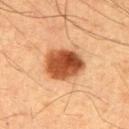The lesion was tiled from a total-body skin photograph and was not biopsied. A roughly 15 mm field-of-view crop from a total-body skin photograph. The lesion's longest dimension is about 5 mm. The lesion is on the arm. Captured under cross-polarized illumination. The subject is a male in their mid- to late 50s.